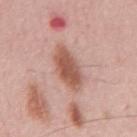The lesion was photographed on a routine skin check and not biopsied; there is no pathology result. A male subject aged around 55. Cropped from a total-body skin-imaging series; the visible field is about 15 mm.